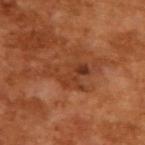Clinical summary:
A region of skin cropped from a whole-body photographic capture, roughly 15 mm wide. A male patient aged 63 to 67. This is a cross-polarized tile.